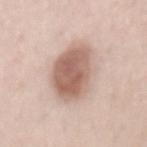Recorded during total-body skin imaging; not selected for excision or biopsy.
The lesion is located on the mid back.
A male subject, approximately 25 years of age.
A roughly 15 mm field-of-view crop from a total-body skin photograph.